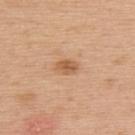This lesion was catalogued during total-body skin photography and was not selected for biopsy.